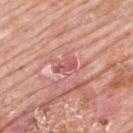Q: Was a biopsy performed?
A: catalogued during a skin exam; not biopsied
Q: What are the patient's age and sex?
A: male, aged 78–82
Q: What is the lesion's diameter?
A: about 2.5 mm
Q: Lesion location?
A: the upper back
Q: What did automated image analysis measure?
A: a border-irregularity rating of about 4.5/10, a within-lesion color-variation index near 0/10, and peripheral color asymmetry of about 0; an automated nevus-likeness rating near 0 out of 100 and a detector confidence of about 95 out of 100 that the crop contains a lesion
Q: What is the imaging modality?
A: total-body-photography crop, ~15 mm field of view
Q: Illumination type?
A: white-light illumination